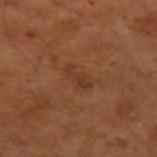notes: total-body-photography surveillance lesion; no biopsy
anatomic site: the left upper arm
imaging modality: ~15 mm crop, total-body skin-cancer survey
subject: male, aged around 55
automated metrics: an average lesion color of about L≈36 a*≈22 b*≈32 (CIELAB), roughly 6 lightness units darker than nearby skin, and a lesion-to-skin contrast of about 5.5 (normalized; higher = more distinct); a border-irregularity index near 5.5/10, a color-variation rating of about 0.5/10, and a peripheral color-asymmetry measure near 0
lighting: cross-polarized illumination
diameter: ~3 mm (longest diameter)